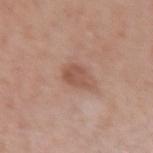{
  "biopsy_status": "not biopsied; imaged during a skin examination",
  "site": "right forearm",
  "lesion_size": {
    "long_diameter_mm_approx": 2.5
  },
  "lighting": "white-light",
  "image": {
    "source": "total-body photography crop",
    "field_of_view_mm": 15
  },
  "patient": {
    "sex": "female",
    "age_approx": 40
  }
}